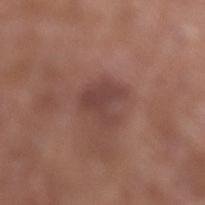workup = catalogued during a skin exam; not biopsied | subject = female, about 80 years old | body site = the left lower leg | image source = ~15 mm tile from a whole-body skin photo | lesion diameter = ≈4 mm.